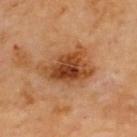On the upper back.
The patient is a male aged 68–72.
About 6 mm across.
A 15 mm crop from a total-body photograph taken for skin-cancer surveillance.